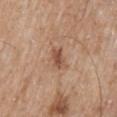Imaged during a routine full-body skin examination; the lesion was not biopsied and no histopathology is available.
This image is a 15 mm lesion crop taken from a total-body photograph.
Located on the mid back.
A male patient, aged around 60.